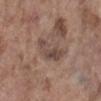Imaged during a routine full-body skin examination; the lesion was not biopsied and no histopathology is available.
Imaged with white-light lighting.
A female patient, aged around 85 or older.
Approximately 5.5 mm at its widest.
Located on the left lower leg.
Automated image analysis of the tile measured an average lesion color of about L≈46 a*≈16 b*≈23 (CIELAB), about 9 CIELAB-L* units darker than the surrounding skin, and a normalized lesion–skin contrast near 7. The analysis additionally found an automated nevus-likeness rating near 0 out of 100.
A 15 mm close-up extracted from a 3D total-body photography capture.